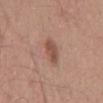Q: Is there a histopathology result?
A: total-body-photography surveillance lesion; no biopsy
Q: What is the imaging modality?
A: total-body-photography crop, ~15 mm field of view
Q: How was the tile lit?
A: white-light illumination
Q: What is the anatomic site?
A: the mid back
Q: What are the patient's age and sex?
A: male, aged approximately 55
Q: Automated lesion metrics?
A: a nevus-likeness score of about 85/100
Q: How large is the lesion?
A: ≈3.5 mm